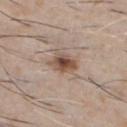Clinical impression: The lesion was tiled from a total-body skin photograph and was not biopsied.